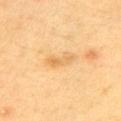Notes:
• notes · no biopsy performed (imaged during a skin exam)
• site · the chest
• size · about 3.5 mm
• subject · male, approximately 60 years of age
• TBP lesion metrics · an area of roughly 3.5 mm², a shape eccentricity near 0.95, and two-axis asymmetry of about 0.35; a lesion color around L≈70 a*≈20 b*≈47 in CIELAB; a classifier nevus-likeness of about 0/100 and a detector confidence of about 100 out of 100 that the crop contains a lesion
• image source · 15 mm crop, total-body photography
• lighting · cross-polarized illumination